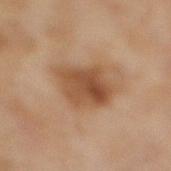{"site": "right lower leg", "lesion_size": {"long_diameter_mm_approx": 6.0}, "image": {"source": "total-body photography crop", "field_of_view_mm": 15}, "automated_metrics": {"area_mm2_approx": 16.0, "shape_asymmetry": 0.2, "cielab_L": 45, "cielab_a": 19, "cielab_b": 31, "vs_skin_darker_L": 11.0, "vs_skin_contrast_norm": 8.5, "border_irregularity_0_10": 2.5, "peripheral_color_asymmetry": 2.0}, "patient": {"sex": "female", "age_approx": 60}}